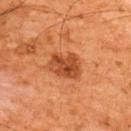Impression: Recorded during total-body skin imaging; not selected for excision or biopsy. Image and clinical context: The recorded lesion diameter is about 3.5 mm. A male patient aged approximately 65. Cropped from a total-body skin-imaging series; the visible field is about 15 mm. Captured under cross-polarized illumination. The lesion-visualizer software estimated an area of roughly 9 mm² and a symmetry-axis asymmetry near 0.2. And it measured a border-irregularity rating of about 2/10. It also reported a nevus-likeness score of about 85/100. Located on the upper back.